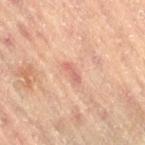This lesion was catalogued during total-body skin photography and was not selected for biopsy. A 15 mm crop from a total-body photograph taken for skin-cancer surveillance. From the left leg. Captured under cross-polarized illumination. A female subject aged approximately 80. The recorded lesion diameter is about 2.5 mm.